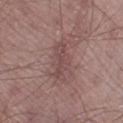follow-up = total-body-photography surveillance lesion; no biopsy
lesion size = ~4.5 mm (longest diameter)
TBP lesion metrics = an automated nevus-likeness rating near 0 out of 100
patient = male, in their mid-60s
site = the left lower leg
imaging modality = ~15 mm crop, total-body skin-cancer survey